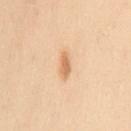The lesion was photographed on a routine skin check and not biopsied; there is no pathology result.